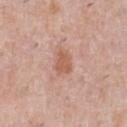Clinical impression:
Captured during whole-body skin photography for melanoma surveillance; the lesion was not biopsied.
Background:
A male patient roughly 40 years of age. This is a white-light tile. The recorded lesion diameter is about 3 mm. Located on the chest. A 15 mm crop from a total-body photograph taken for skin-cancer surveillance. Automated image analysis of the tile measured a lesion area of about 5 mm² and a shape-asymmetry score of about 0.2 (0 = symmetric). And it measured an average lesion color of about L≈58 a*≈23 b*≈31 (CIELAB) and a normalized lesion–skin contrast near 7. The software also gave a border-irregularity rating of about 1.5/10.